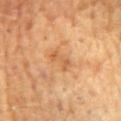Impression: Imaged during a routine full-body skin examination; the lesion was not biopsied and no histopathology is available. Context: The tile uses cross-polarized illumination. A 15 mm crop from a total-body photograph taken for skin-cancer surveillance. A male patient about 60 years old. Automated image analysis of the tile measured a lesion area of about 3.5 mm² and an outline eccentricity of about 0.85 (0 = round, 1 = elongated). The software also gave a border-irregularity index near 5.5/10, a within-lesion color-variation index near 1/10, and radial color variation of about 0. Located on the back.